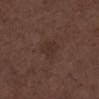<case>
  <biopsy_status>not biopsied; imaged during a skin examination</biopsy_status>
  <patient>
    <sex>male</sex>
    <age_approx>70</age_approx>
  </patient>
  <lesion_size>
    <long_diameter_mm_approx>3.5</long_diameter_mm_approx>
  </lesion_size>
  <image>
    <source>total-body photography crop</source>
    <field_of_view_mm>15</field_of_view_mm>
  </image>
  <site>leg</site>
  <lighting>white-light</lighting>
</case>